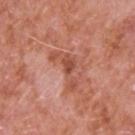site: the upper back; lesion size: ≈5 mm; image source: ~15 mm tile from a whole-body skin photo; subject: male, aged 63–67.